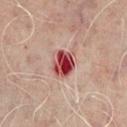The lesion was tiled from a total-body skin photograph and was not biopsied. A region of skin cropped from a whole-body photographic capture, roughly 15 mm wide. From the mid back. The lesion's longest dimension is about 3.5 mm. A male subject roughly 70 years of age.